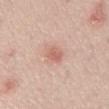Located on the abdomen. A 15 mm crop from a total-body photograph taken for skin-cancer surveillance. Automated image analysis of the tile measured a lesion area of about 4 mm² and an outline eccentricity of about 0.7 (0 = round, 1 = elongated). It also reported a normalized lesion–skin contrast near 6. The software also gave a border-irregularity rating of about 2/10, a within-lesion color-variation index near 3.5/10, and a peripheral color-asymmetry measure near 1.5. It also reported a lesion-detection confidence of about 100/100. A male subject aged 68–72. The tile uses white-light illumination.